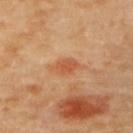  biopsy_status: not biopsied; imaged during a skin examination
  site: upper back
  automated_metrics:
    color_variation_0_10: 1.0
    peripheral_color_asymmetry: 0.5
    nevus_likeness_0_100: 90
    lesion_detection_confidence_0_100: 100
  image:
    source: total-body photography crop
    field_of_view_mm: 15
  patient:
    sex: female
    age_approx: 60